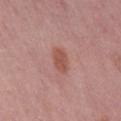follow-up=imaged on a skin check; not biopsied | tile lighting=white-light illumination | site=the back | image source=total-body-photography crop, ~15 mm field of view | subject=male, aged around 60.